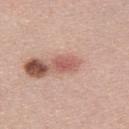Findings:
- workup · catalogued during a skin exam; not biopsied
- TBP lesion metrics · a lesion color around L≈57 a*≈26 b*≈25 in CIELAB, roughly 11 lightness units darker than nearby skin, and a normalized border contrast of about 7.5; a border-irregularity index near 2/10 and a within-lesion color-variation index near 1.5/10; an automated nevus-likeness rating near 65 out of 100 and a detector confidence of about 100 out of 100 that the crop contains a lesion
- acquisition · ~15 mm tile from a whole-body skin photo
- lesion diameter · ≈3 mm
- lighting · white-light illumination
- patient · male, about 30 years old